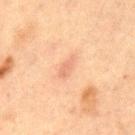Captured during whole-body skin photography for melanoma surveillance; the lesion was not biopsied.
On the front of the torso.
Measured at roughly 3 mm in maximum diameter.
The patient is a male aged approximately 65.
Automated image analysis of the tile measured a lesion area of about 4 mm² and an eccentricity of roughly 0.9. And it measured a lesion color around L≈56 a*≈20 b*≈29 in CIELAB, about 6 CIELAB-L* units darker than the surrounding skin, and a normalized border contrast of about 4.5. And it measured a border-irregularity index near 3/10, a within-lesion color-variation index near 1/10, and a peripheral color-asymmetry measure near 0.5. The software also gave an automated nevus-likeness rating near 0 out of 100 and a lesion-detection confidence of about 100/100.
Cropped from a whole-body photographic skin survey; the tile spans about 15 mm.